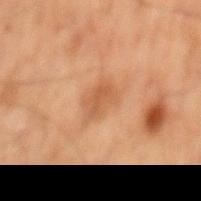Assessment: Captured during whole-body skin photography for melanoma surveillance; the lesion was not biopsied. Clinical summary: A male subject in their 70s. On the mid back. A close-up tile cropped from a whole-body skin photograph, about 15 mm across. About 4 mm across.